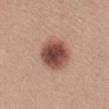Case summary:
* follow-up · catalogued during a skin exam; not biopsied
* image source · ~15 mm crop, total-body skin-cancer survey
* location · the mid back
* subject · female, roughly 35 years of age
* size · about 4.5 mm